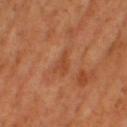The lesion was photographed on a routine skin check and not biopsied; there is no pathology result. This is a cross-polarized tile. An algorithmic analysis of the crop reported a mean CIELAB color near L≈48 a*≈28 b*≈38, a lesion–skin lightness drop of about 7, and a normalized border contrast of about 5.5. It also reported a border-irregularity rating of about 4/10. It also reported a nevus-likeness score of about 0/100 and lesion-presence confidence of about 100/100. A lesion tile, about 15 mm wide, cut from a 3D total-body photograph. Longest diameter approximately 2.5 mm. The lesion is located on the right thigh. A female patient, aged 53 to 57.